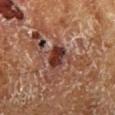This lesion was catalogued during total-body skin photography and was not selected for biopsy.
From the right lower leg.
Imaged with cross-polarized lighting.
A 15 mm close-up extracted from a 3D total-body photography capture.
Longest diameter approximately 3 mm.
The total-body-photography lesion software estimated a lesion area of about 6.5 mm², an eccentricity of roughly 0.6, and a shape-asymmetry score of about 0.25 (0 = symmetric). And it measured a mean CIELAB color near L≈35 a*≈24 b*≈24 and a lesion-to-skin contrast of about 11 (normalized; higher = more distinct). It also reported a classifier nevus-likeness of about 0/100 and a detector confidence of about 100 out of 100 that the crop contains a lesion.
The subject is a male about 70 years old.